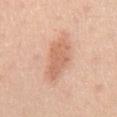Findings:
* follow-up: total-body-photography surveillance lesion; no biopsy
* imaging modality: ~15 mm crop, total-body skin-cancer survey
* patient: male, in their mid-40s
* image-analysis metrics: a mean CIELAB color near L≈65 a*≈23 b*≈32 and a lesion-to-skin contrast of about 6.5 (normalized; higher = more distinct); an automated nevus-likeness rating near 20 out of 100 and a lesion-detection confidence of about 100/100
* site: the chest